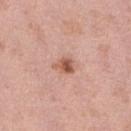follow-up = no biopsy performed (imaged during a skin exam); image = 15 mm crop, total-body photography; subject = female, approximately 40 years of age; lighting = white-light illumination; lesion diameter = about 2.5 mm; site = the right lower leg.